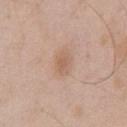Case summary:
- notes · total-body-photography surveillance lesion; no biopsy
- image-analysis metrics · a lesion color around L≈60 a*≈18 b*≈30 in CIELAB and a normalized lesion–skin contrast near 5.5; a nevus-likeness score of about 5/100 and a lesion-detection confidence of about 100/100
- location · the chest
- image source · ~15 mm crop, total-body skin-cancer survey
- patient · male, about 50 years old
- lesion size · ~3 mm (longest diameter)